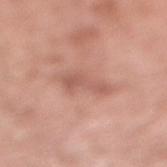A 15 mm crop from a total-body photograph taken for skin-cancer surveillance. From the right lower leg. A male subject, approximately 80 years of age. The lesion's longest dimension is about 4 mm. The tile uses white-light illumination.